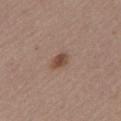notes = total-body-photography surveillance lesion; no biopsy.